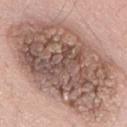Impression:
Imaged during a routine full-body skin examination; the lesion was not biopsied and no histopathology is available.
Image and clinical context:
The lesion's longest dimension is about 14 mm. Located on the mid back. A male patient aged approximately 60. The tile uses white-light illumination. A region of skin cropped from a whole-body photographic capture, roughly 15 mm wide.